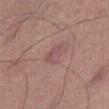{"biopsy_status": "not biopsied; imaged during a skin examination", "image": {"source": "total-body photography crop", "field_of_view_mm": 15}, "patient": {"sex": "female", "age_approx": 45}, "lighting": "white-light", "site": "right thigh"}